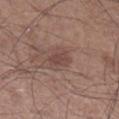Context: This image is a 15 mm lesion crop taken from a total-body photograph. A male patient, aged 58 to 62. The lesion is located on the right lower leg.